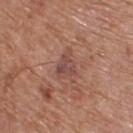biopsy status: catalogued during a skin exam; not biopsied
lesion diameter: ~3 mm (longest diameter)
anatomic site: the upper back
automated lesion analysis: a lesion area of about 5 mm² and a shape-asymmetry score of about 0.45 (0 = symmetric)
acquisition: total-body-photography crop, ~15 mm field of view
subject: male, roughly 65 years of age